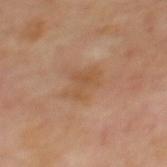| key | value |
|---|---|
| tile lighting | cross-polarized |
| image | ~15 mm tile from a whole-body skin photo |
| patient | male, in their 70s |
| location | the mid back |